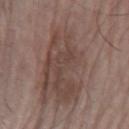Context:
A male patient, aged around 65. A 15 mm close-up tile from a total-body photography series done for melanoma screening. Imaged with white-light lighting. The lesion is on the arm. Measured at roughly 15 mm in maximum diameter.